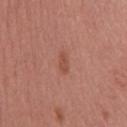Assessment:
Captured during whole-body skin photography for melanoma surveillance; the lesion was not biopsied.
Image and clinical context:
Cropped from a total-body skin-imaging series; the visible field is about 15 mm. The lesion is on the upper back. The tile uses white-light illumination. Longest diameter approximately 2.5 mm. The subject is a male about 45 years old.